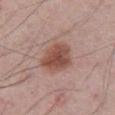A male subject aged around 75. About 4.5 mm across. Cropped from a total-body skin-imaging series; the visible field is about 15 mm. Located on the abdomen. The tile uses white-light illumination.